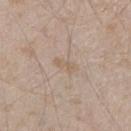biopsy status = total-body-photography surveillance lesion; no biopsy
image source = 15 mm crop, total-body photography
lesion diameter = ≈2.5 mm
tile lighting = white-light illumination
subject = male, roughly 45 years of age
anatomic site = the right lower leg